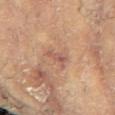This is a cross-polarized tile.
From the right arm.
Automated tile analysis of the lesion measured two-axis asymmetry of about 0.45. And it measured a mean CIELAB color near L≈49 a*≈21 b*≈25, roughly 7 lightness units darker than nearby skin, and a lesion-to-skin contrast of about 6 (normalized; higher = more distinct). It also reported a classifier nevus-likeness of about 0/100 and lesion-presence confidence of about 85/100.
A 15 mm crop from a total-body photograph taken for skin-cancer surveillance.
A female subject approximately 80 years of age.
Measured at roughly 2.5 mm in maximum diameter.